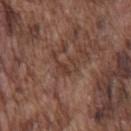workup: catalogued during a skin exam; not biopsied
tile lighting: white-light illumination
image source: ~15 mm crop, total-body skin-cancer survey
patient: male, in their mid- to late 70s
body site: the chest
TBP lesion metrics: a lesion area of about 4.5 mm², an outline eccentricity of about 0.75 (0 = round, 1 = elongated), and a shape-asymmetry score of about 0.7 (0 = symmetric); a border-irregularity rating of about 9/10, a color-variation rating of about 2/10, and peripheral color asymmetry of about 1; an automated nevus-likeness rating near 0 out of 100 and a lesion-detection confidence of about 85/100
lesion diameter: ≈3.5 mm